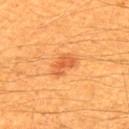Assessment: No biopsy was performed on this lesion — it was imaged during a full skin examination and was not determined to be concerning. Acquisition and patient details: A 15 mm close-up tile from a total-body photography series done for melanoma screening. A male patient approximately 60 years of age. Imaged with cross-polarized lighting. The lesion is on the upper back.